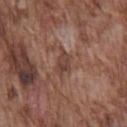Assessment: Captured during whole-body skin photography for melanoma surveillance; the lesion was not biopsied. Image and clinical context: A 15 mm close-up extracted from a 3D total-body photography capture. The lesion is located on the chest. A male subject, in their mid- to late 70s.